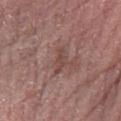Clinical impression: No biopsy was performed on this lesion — it was imaged during a full skin examination and was not determined to be concerning. Clinical summary: From the right forearm. A lesion tile, about 15 mm wide, cut from a 3D total-body photograph. The subject is a female about 65 years old.